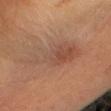{"biopsy_status": "not biopsied; imaged during a skin examination"}